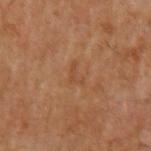Notes:
- workup — imaged on a skin check; not biopsied
- tile lighting — cross-polarized illumination
- lesion size — ~2.5 mm (longest diameter)
- patient — male, aged approximately 60
- location — the arm
- image-analysis metrics — an area of roughly 2.5 mm²; an average lesion color of about L≈44 a*≈20 b*≈33 (CIELAB) and roughly 5 lightness units darker than nearby skin; an automated nevus-likeness rating near 0 out of 100
- imaging modality — ~15 mm crop, total-body skin-cancer survey